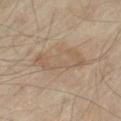No biopsy was performed on this lesion — it was imaged during a full skin examination and was not determined to be concerning. The lesion's longest dimension is about 6.5 mm. This image is a 15 mm lesion crop taken from a total-body photograph. An algorithmic analysis of the crop reported an outline eccentricity of about 0.9 (0 = round, 1 = elongated) and a shape-asymmetry score of about 0.35 (0 = symmetric). And it measured a mean CIELAB color near L≈55 a*≈13 b*≈29, about 6 CIELAB-L* units darker than the surrounding skin, and a normalized border contrast of about 5. The analysis additionally found a border-irregularity index near 4.5/10 and a within-lesion color-variation index near 2.5/10. The subject is aged around 55. The lesion is located on the left thigh.